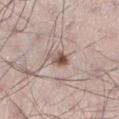<lesion>
  <image>
    <source>total-body photography crop</source>
    <field_of_view_mm>15</field_of_view_mm>
  </image>
  <site>left lower leg</site>
  <lighting>white-light</lighting>
  <automated_metrics>
    <eccentricity>0.7</eccentricity>
    <shape_asymmetry>0.25</shape_asymmetry>
    <cielab_L>54</cielab_L>
    <cielab_a>17</cielab_a>
    <cielab_b>23</cielab_b>
    <vs_skin_darker_L>13.0</vs_skin_darker_L>
    <vs_skin_contrast_norm>9.0</vs_skin_contrast_norm>
    <color_variation_0_10>5.5</color_variation_0_10>
    <peripheral_color_asymmetry>2.0</peripheral_color_asymmetry>
  </automated_metrics>
  <patient>
    <sex>male</sex>
    <age_approx>35</age_approx>
  </patient>
  <lesion_size>
    <long_diameter_mm_approx>2.5</long_diameter_mm_approx>
  </lesion_size>
</lesion>